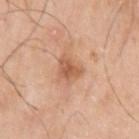Clinical impression: Recorded during total-body skin imaging; not selected for excision or biopsy. Image and clinical context: A 15 mm crop from a total-body photograph taken for skin-cancer surveillance. On the left upper arm. A male patient aged 63–67. Measured at roughly 3 mm in maximum diameter. Imaged with white-light lighting.